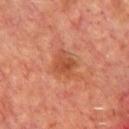Captured during whole-body skin photography for melanoma surveillance; the lesion was not biopsied. An algorithmic analysis of the crop reported a border-irregularity rating of about 3/10 and radial color variation of about 1. And it measured a classifier nevus-likeness of about 5/100. Captured under cross-polarized illumination. A male subject aged approximately 65. On the back. About 3 mm across. This image is a 15 mm lesion crop taken from a total-body photograph.